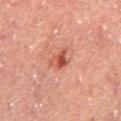Clinical impression: The lesion was photographed on a routine skin check and not biopsied; there is no pathology result. Acquisition and patient details: The lesion is located on the back. A close-up tile cropped from a whole-body skin photograph, about 15 mm across. A male subject, in their 60s. About 2.5 mm across.